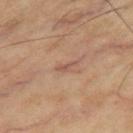biopsy_status: not biopsied; imaged during a skin examination
automated_metrics:
  area_mm2_approx: 2.0
  eccentricity: 0.9
  shape_asymmetry: 0.35
  vs_skin_darker_L: 8.0
  nevus_likeness_0_100: 0
  lesion_detection_confidence_0_100: 95
image:
  source: total-body photography crop
  field_of_view_mm: 15
patient:
  sex: male
  age_approx: 65
site: leg
lighting: cross-polarized
lesion_size:
  long_diameter_mm_approx: 2.5